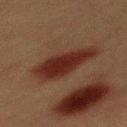Assessment:
Part of a total-body skin-imaging series; this lesion was reviewed on a skin check and was not flagged for biopsy.
Image and clinical context:
About 6.5 mm across. The lesion is on the mid back. The tile uses cross-polarized illumination. This image is a 15 mm lesion crop taken from a total-body photograph. The subject is a male aged 38–42.